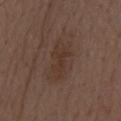biopsy status: imaged on a skin check; not biopsied
anatomic site: the back
subject: male, about 70 years old
imaging modality: ~15 mm tile from a whole-body skin photo
image-analysis metrics: a border-irregularity index near 3.5/10, a color-variation rating of about 2.5/10, and peripheral color asymmetry of about 1; an automated nevus-likeness rating near 5 out of 100 and a detector confidence of about 100 out of 100 that the crop contains a lesion